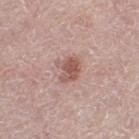Q: Is there a histopathology result?
A: catalogued during a skin exam; not biopsied
Q: What did automated image analysis measure?
A: an outline eccentricity of about 0.55 (0 = round, 1 = elongated) and a symmetry-axis asymmetry near 0.3; a mean CIELAB color near L≈55 a*≈21 b*≈25, about 11 CIELAB-L* units darker than the surrounding skin, and a normalized lesion–skin contrast near 7.5
Q: Lesion location?
A: the left thigh
Q: What is the imaging modality?
A: total-body-photography crop, ~15 mm field of view
Q: How was the tile lit?
A: white-light
Q: What are the patient's age and sex?
A: female, about 65 years old
Q: What is the lesion's diameter?
A: ≈3 mm